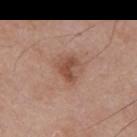- notes — total-body-photography surveillance lesion; no biopsy
- diameter — ~3 mm (longest diameter)
- lighting — white-light illumination
- patient — male, in their 50s
- location — the front of the torso
- image source — 15 mm crop, total-body photography